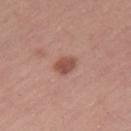  biopsy_status: not biopsied; imaged during a skin examination
  lesion_size:
    long_diameter_mm_approx: 3.0
  automated_metrics:
    lesion_detection_confidence_0_100: 100
  patient:
    sex: female
    age_approx: 50
  image:
    source: total-body photography crop
    field_of_view_mm: 15
  site: right thigh